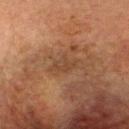The lesion was photographed on a routine skin check and not biopsied; there is no pathology result.
Captured under cross-polarized illumination.
This image is a 15 mm lesion crop taken from a total-body photograph.
A male subject in their mid- to late 60s.
Approximately 3 mm at its widest.
The lesion is on the head or neck.
The total-body-photography lesion software estimated a lesion area of about 4.5 mm², an eccentricity of roughly 0.7, and two-axis asymmetry of about 0.35. The analysis additionally found an average lesion color of about L≈36 a*≈17 b*≈27 (CIELAB), about 5 CIELAB-L* units darker than the surrounding skin, and a normalized lesion–skin contrast near 4.5. The analysis additionally found an automated nevus-likeness rating near 0 out of 100 and a lesion-detection confidence of about 100/100.